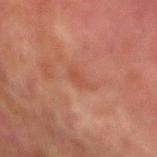Impression:
No biopsy was performed on this lesion — it was imaged during a full skin examination and was not determined to be concerning.
Clinical summary:
The tile uses cross-polarized illumination. The subject is a male aged 73–77. A lesion tile, about 15 mm wide, cut from a 3D total-body photograph. The lesion is on the left forearm. The lesion's longest dimension is about 3 mm.